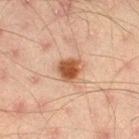Clinical impression: Recorded during total-body skin imaging; not selected for excision or biopsy. Acquisition and patient details: Cropped from a total-body skin-imaging series; the visible field is about 15 mm. From the right thigh. Imaged with cross-polarized lighting. A male subject aged 43 to 47.